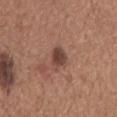Imaged during a routine full-body skin examination; the lesion was not biopsied and no histopathology is available. A region of skin cropped from a whole-body photographic capture, roughly 15 mm wide. A male patient, roughly 65 years of age. On the mid back.